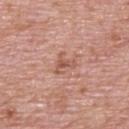Acquisition and patient details:
A male subject in their 70s. Located on the upper back. A 15 mm close-up extracted from a 3D total-body photography capture.